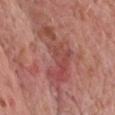Acquisition and patient details:
This is a white-light tile. A male subject, roughly 60 years of age. A close-up tile cropped from a whole-body skin photograph, about 15 mm across. On the chest.